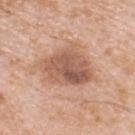<record>
  <image>
    <source>total-body photography crop</source>
    <field_of_view_mm>15</field_of_view_mm>
  </image>
  <lesion_size>
    <long_diameter_mm_approx>7.0</long_diameter_mm_approx>
  </lesion_size>
  <lighting>white-light</lighting>
  <automated_metrics>
    <shape_asymmetry>0.3</shape_asymmetry>
    <cielab_L>57</cielab_L>
    <cielab_a>21</cielab_a>
    <cielab_b>30</cielab_b>
    <vs_skin_darker_L>12.0</vs_skin_darker_L>
  </automated_metrics>
  <site>upper back</site>
  <patient>
    <sex>male</sex>
    <age_approx>80</age_approx>
  </patient>
</record>